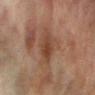Impression:
The lesion was photographed on a routine skin check and not biopsied; there is no pathology result.
Acquisition and patient details:
On the right forearm. Automated image analysis of the tile measured about 7 CIELAB-L* units darker than the surrounding skin. It also reported a border-irregularity rating of about 2/10, a within-lesion color-variation index near 3.5/10, and radial color variation of about 1. A close-up tile cropped from a whole-body skin photograph, about 15 mm across. A female patient, roughly 80 years of age. The tile uses cross-polarized illumination.